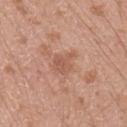The lesion's longest dimension is about 2.5 mm. The patient is a male aged 18–22. Located on the arm. The lesion-visualizer software estimated a border-irregularity rating of about 4/10, a within-lesion color-variation index near 2/10, and a peripheral color-asymmetry measure near 0.5. The analysis additionally found a nevus-likeness score of about 0/100 and a lesion-detection confidence of about 100/100. This image is a 15 mm lesion crop taken from a total-body photograph.